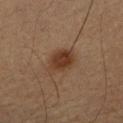notes = no biopsy performed (imaged during a skin exam)
lighting = cross-polarized
body site = the left forearm
image = 15 mm crop, total-body photography
lesion diameter = ≈4 mm
patient = male, in their mid- to late 50s